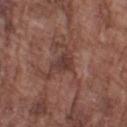The lesion-visualizer software estimated an average lesion color of about L≈38 a*≈20 b*≈22 (CIELAB), a lesion–skin lightness drop of about 8, and a lesion-to-skin contrast of about 7 (normalized; higher = more distinct). And it measured an automated nevus-likeness rating near 0 out of 100 and a detector confidence of about 100 out of 100 that the crop contains a lesion. The lesion is located on the left upper arm. Approximately 3 mm at its widest. The tile uses white-light illumination. A region of skin cropped from a whole-body photographic capture, roughly 15 mm wide. The subject is a male about 75 years old.